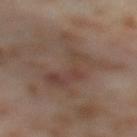Imaged during a routine full-body skin examination; the lesion was not biopsied and no histopathology is available. The tile uses cross-polarized illumination. The lesion's longest dimension is about 9 mm. The patient is a female in their mid- to late 50s. The lesion is on the leg. A 15 mm close-up extracted from a 3D total-body photography capture. Automated tile analysis of the lesion measured a mean CIELAB color near L≈43 a*≈15 b*≈23.